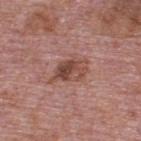Notes:
- biopsy status: total-body-photography surveillance lesion; no biopsy
- anatomic site: the upper back
- acquisition: total-body-photography crop, ~15 mm field of view
- patient: male, in their mid- to late 70s
- lesion diameter: about 4.5 mm